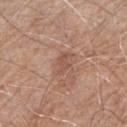No biopsy was performed on this lesion — it was imaged during a full skin examination and was not determined to be concerning.
Captured under white-light illumination.
The lesion is located on the right upper arm.
Measured at roughly 3 mm in maximum diameter.
A male subject, aged 58–62.
A region of skin cropped from a whole-body photographic capture, roughly 15 mm wide.